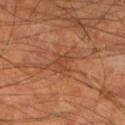<record>
  <biopsy_status>not biopsied; imaged during a skin examination</biopsy_status>
  <automated_metrics>
    <cielab_L>39</cielab_L>
    <cielab_a>23</cielab_a>
    <cielab_b>31</cielab_b>
    <color_variation_0_10>1.5</color_variation_0_10>
    <peripheral_color_asymmetry>0.5</peripheral_color_asymmetry>
    <nevus_likeness_0_100>0</nevus_likeness_0_100>
    <lesion_detection_confidence_0_100>70</lesion_detection_confidence_0_100>
  </automated_metrics>
  <lighting>cross-polarized</lighting>
  <image>
    <source>total-body photography crop</source>
    <field_of_view_mm>15</field_of_view_mm>
  </image>
  <site>left lower leg</site>
  <patient>
    <sex>male</sex>
    <age_approx>65</age_approx>
  </patient>
</record>